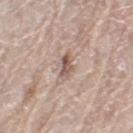lighting: white-light
site: left thigh
automated_metrics:
  border_irregularity_0_10: 4.0
  color_variation_0_10: 1.5
  peripheral_color_asymmetry: 0.5
  nevus_likeness_0_100: 0
patient:
  sex: female
  age_approx: 60
lesion_size:
  long_diameter_mm_approx: 3.5
image:
  source: total-body photography crop
  field_of_view_mm: 15